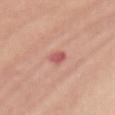notes = no biopsy performed (imaged during a skin exam)
image-analysis metrics = an area of roughly 3 mm², a shape eccentricity near 0.85, and a symmetry-axis asymmetry near 0.25; a lesion–skin lightness drop of about 11 and a normalized border contrast of about 7.5
illumination = white-light illumination
lesion size = ~2.5 mm (longest diameter)
subject = female, aged 48–52
body site = the back
acquisition = ~15 mm tile from a whole-body skin photo